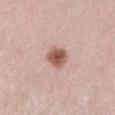Q: Is there a histopathology result?
A: imaged on a skin check; not biopsied
Q: Illumination type?
A: white-light illumination
Q: What is the imaging modality?
A: ~15 mm crop, total-body skin-cancer survey
Q: Patient demographics?
A: male, approximately 30 years of age